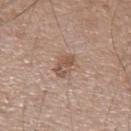This lesion was catalogued during total-body skin photography and was not selected for biopsy.
From the left forearm.
A male patient aged 53–57.
Cropped from a total-body skin-imaging series; the visible field is about 15 mm.
This is a white-light tile.
The recorded lesion diameter is about 3 mm.